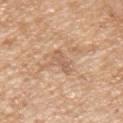biopsy status: no biopsy performed (imaged during a skin exam); subject: male, aged approximately 60; imaging modality: 15 mm crop, total-body photography; lighting: white-light illumination; site: the left upper arm; lesion size: ~3 mm (longest diameter).